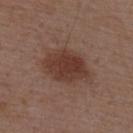notes: catalogued during a skin exam; not biopsied | acquisition: total-body-photography crop, ~15 mm field of view | lesion size: ≈6 mm | subject: male, aged approximately 50 | site: the upper back | lighting: white-light | image-analysis metrics: a lesion-detection confidence of about 100/100.